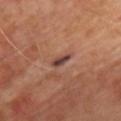workup: imaged on a skin check; not biopsied | anatomic site: the chest | lesion diameter: about 2.5 mm | subject: male, approximately 65 years of age | image source: ~15 mm crop, total-body skin-cancer survey | tile lighting: cross-polarized illumination.